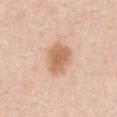  biopsy_status: not biopsied; imaged during a skin examination
  site: abdomen
  lesion_size:
    long_diameter_mm_approx: 4.0
  image:
    source: total-body photography crop
    field_of_view_mm: 15
  patient:
    sex: male
    age_approx: 50
  lighting: white-light
  automated_metrics:
    area_mm2_approx: 10.0
    eccentricity: 0.65
    shape_asymmetry: 0.2
    cielab_L: 65
    cielab_a: 21
    cielab_b: 33
    vs_skin_darker_L: 12.0
    vs_skin_contrast_norm: 8.0
    peripheral_color_asymmetry: 0.5
    lesion_detection_confidence_0_100: 100